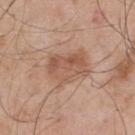| key | value |
|---|---|
| notes | total-body-photography surveillance lesion; no biopsy |
| acquisition | ~15 mm crop, total-body skin-cancer survey |
| illumination | white-light |
| TBP lesion metrics | an area of roughly 14 mm², a shape eccentricity near 0.65, and a shape-asymmetry score of about 0.25 (0 = symmetric); a mean CIELAB color near L≈55 a*≈21 b*≈30, roughly 9 lightness units darker than nearby skin, and a normalized lesion–skin contrast near 6.5; a border-irregularity index near 3/10; a classifier nevus-likeness of about 15/100 |
| location | the back |
| diameter | ≈5 mm |
| subject | male, in their mid- to late 50s |